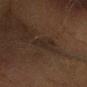Captured during whole-body skin photography for melanoma surveillance; the lesion was not biopsied. Cropped from a total-body skin-imaging series; the visible field is about 15 mm. Imaged with cross-polarized lighting. The lesion is on the head or neck. A male subject, approximately 65 years of age. Measured at roughly 3.5 mm in maximum diameter.